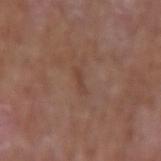Case summary:
- notes: no biopsy performed (imaged during a skin exam)
- automated lesion analysis: an outline eccentricity of about 0.95 (0 = round, 1 = elongated) and a symmetry-axis asymmetry near 0.45; a mean CIELAB color near L≈42 a*≈20 b*≈26, roughly 6 lightness units darker than nearby skin, and a normalized border contrast of about 5; border irregularity of about 5 on a 0–10 scale, internal color variation of about 0 on a 0–10 scale, and a peripheral color-asymmetry measure near 0; a nevus-likeness score of about 0/100 and a detector confidence of about 90 out of 100 that the crop contains a lesion
- imaging modality: ~15 mm crop, total-body skin-cancer survey
- body site: the right forearm
- patient: male, roughly 65 years of age